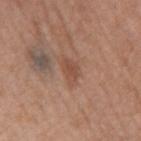The lesion was photographed on a routine skin check and not biopsied; there is no pathology result.
A male subject aged approximately 75.
Approximately 3 mm at its widest.
A roughly 15 mm field-of-view crop from a total-body skin photograph.
This is a white-light tile.
The lesion is located on the mid back.